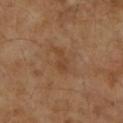This lesion was catalogued during total-body skin photography and was not selected for biopsy.
A 15 mm crop from a total-body photograph taken for skin-cancer surveillance.
A female subject, roughly 70 years of age.
The lesion is located on the left forearm.